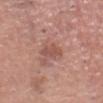Q: Was this lesion biopsied?
A: catalogued during a skin exam; not biopsied
Q: What is the imaging modality?
A: ~15 mm crop, total-body skin-cancer survey
Q: What are the patient's age and sex?
A: male, in their mid- to late 50s
Q: How was the tile lit?
A: white-light